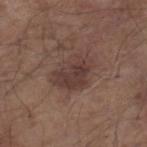{"biopsy_status": "not biopsied; imaged during a skin examination", "image": {"source": "total-body photography crop", "field_of_view_mm": 15}, "site": "right lower leg", "patient": {"sex": "male", "age_approx": 80}}